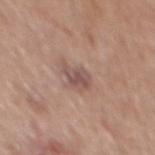Q: Was this lesion biopsied?
A: no biopsy performed (imaged during a skin exam)
Q: Who is the patient?
A: male, aged 68 to 72
Q: Lesion size?
A: ~3 mm (longest diameter)
Q: Automated lesion metrics?
A: an area of roughly 4.5 mm² and two-axis asymmetry of about 0.35; an automated nevus-likeness rating near 0 out of 100 and a lesion-detection confidence of about 100/100
Q: Where on the body is the lesion?
A: the mid back
Q: How was this image acquired?
A: 15 mm crop, total-body photography
Q: Illumination type?
A: white-light illumination